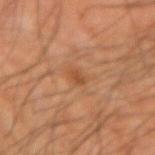Recorded during total-body skin imaging; not selected for excision or biopsy.
From the left forearm.
A male subject, aged approximately 60.
Cropped from a whole-body photographic skin survey; the tile spans about 15 mm.
Captured under cross-polarized illumination.
Longest diameter approximately 2 mm.